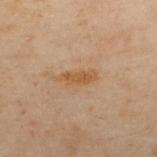Part of a total-body skin-imaging series; this lesion was reviewed on a skin check and was not flagged for biopsy.
The recorded lesion diameter is about 4 mm.
The lesion-visualizer software estimated an area of roughly 5 mm², a shape eccentricity near 0.9, and a shape-asymmetry score of about 0.3 (0 = symmetric). The software also gave about 6 CIELAB-L* units darker than the surrounding skin and a lesion-to-skin contrast of about 7.5 (normalized; higher = more distinct). The software also gave an automated nevus-likeness rating near 60 out of 100 and a detector confidence of about 100 out of 100 that the crop contains a lesion.
A male subject aged 48–52.
The lesion is located on the upper back.
A roughly 15 mm field-of-view crop from a total-body skin photograph.